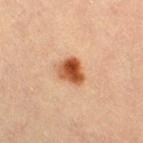<tbp_lesion>
  <biopsy_status>not biopsied; imaged during a skin examination</biopsy_status>
  <automated_metrics>
    <area_mm2_approx>7.0</area_mm2_approx>
    <shape_asymmetry>0.25</shape_asymmetry>
    <color_variation_0_10>8.5</color_variation_0_10>
    <peripheral_color_asymmetry>2.5</peripheral_color_asymmetry>
    <nevus_likeness_0_100>100</nevus_likeness_0_100>
    <lesion_detection_confidence_0_100>100</lesion_detection_confidence_0_100>
  </automated_metrics>
  <site>right thigh</site>
  <lesion_size>
    <long_diameter_mm_approx>3.5</long_diameter_mm_approx>
  </lesion_size>
  <image>
    <source>total-body photography crop</source>
    <field_of_view_mm>15</field_of_view_mm>
  </image>
  <lighting>cross-polarized</lighting>
  <patient>
    <sex>female</sex>
    <age_approx>30</age_approx>
  </patient>
</tbp_lesion>